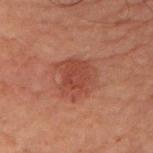Imaged during a routine full-body skin examination; the lesion was not biopsied and no histopathology is available.
A male patient aged 68 to 72.
Cropped from a whole-body photographic skin survey; the tile spans about 15 mm.
Imaged with cross-polarized lighting.
The total-body-photography lesion software estimated a lesion color around L≈34 a*≈21 b*≈24 in CIELAB, a lesion–skin lightness drop of about 7, and a lesion-to-skin contrast of about 6 (normalized; higher = more distinct). It also reported a border-irregularity index near 3.5/10 and a within-lesion color-variation index near 2.5/10.
Longest diameter approximately 4 mm.
From the arm.